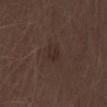{
  "biopsy_status": "not biopsied; imaged during a skin examination",
  "lesion_size": {
    "long_diameter_mm_approx": 2.5
  },
  "image": {
    "source": "total-body photography crop",
    "field_of_view_mm": 15
  },
  "patient": {
    "sex": "male",
    "age_approx": 70
  },
  "site": "left forearm",
  "automated_metrics": {
    "nevus_likeness_0_100": 5,
    "lesion_detection_confidence_0_100": 100
  }
}